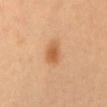Q: Automated lesion metrics?
A: a shape eccentricity near 0.75 and a symmetry-axis asymmetry near 0.3; a border-irregularity rating of about 2.5/10, a within-lesion color-variation index near 2.5/10, and radial color variation of about 0.5
Q: How was the tile lit?
A: cross-polarized illumination
Q: Lesion size?
A: about 3 mm
Q: Lesion location?
A: the mid back
Q: What is the imaging modality?
A: total-body-photography crop, ~15 mm field of view
Q: What are the patient's age and sex?
A: female, about 60 years old